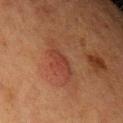Clinical impression: Captured during whole-body skin photography for melanoma surveillance; the lesion was not biopsied. Acquisition and patient details: A region of skin cropped from a whole-body photographic capture, roughly 15 mm wide. The subject is a female aged 48–52. Automated tile analysis of the lesion measured a footprint of about 4.5 mm², an outline eccentricity of about 0.9 (0 = round, 1 = elongated), and a symmetry-axis asymmetry near 0.3. The analysis additionally found an average lesion color of about L≈33 a*≈23 b*≈25 (CIELAB), about 5 CIELAB-L* units darker than the surrounding skin, and a lesion-to-skin contrast of about 5.5 (normalized; higher = more distinct). It also reported an automated nevus-likeness rating near 30 out of 100. The lesion is located on the left forearm.